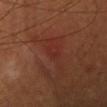workup: total-body-photography surveillance lesion; no biopsy | subject: female, approximately 50 years of age | image source: total-body-photography crop, ~15 mm field of view | location: the head or neck | automated metrics: an average lesion color of about L≈31 a*≈28 b*≈27 (CIELAB), a lesion–skin lightness drop of about 4, and a normalized border contrast of about 4 | tile lighting: cross-polarized | lesion diameter: about 2.5 mm.